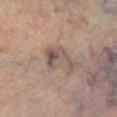Clinical impression:
This lesion was catalogued during total-body skin photography and was not selected for biopsy.
Context:
A male subject roughly 70 years of age. This image is a 15 mm lesion crop taken from a total-body photograph. Located on the right lower leg.